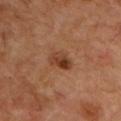biopsy status — imaged on a skin check; not biopsied
acquisition — ~15 mm tile from a whole-body skin photo
size — ≈3 mm
anatomic site — the chest
subject — male, about 50 years old
automated lesion analysis — a mean CIELAB color near L≈39 a*≈23 b*≈32 and a lesion–skin lightness drop of about 10
illumination — cross-polarized